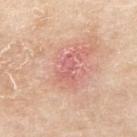Background:
The lesion is located on the right upper arm. Captured under white-light illumination. A female subject aged approximately 70. A 15 mm close-up tile from a total-body photography series done for melanoma screening.